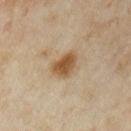Q: Was a biopsy performed?
A: catalogued during a skin exam; not biopsied
Q: What lighting was used for the tile?
A: cross-polarized
Q: Patient demographics?
A: female, aged 33 to 37
Q: How was this image acquired?
A: total-body-photography crop, ~15 mm field of view
Q: Where on the body is the lesion?
A: the left upper arm